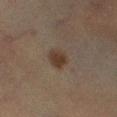Captured during whole-body skin photography for melanoma surveillance; the lesion was not biopsied.
The patient is a male aged 58 to 62.
Captured under cross-polarized illumination.
The lesion is on the right lower leg.
A roughly 15 mm field-of-view crop from a total-body skin photograph.
About 2.5 mm across.